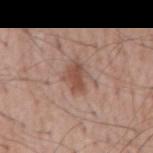Case summary:
* notes · catalogued during a skin exam; not biopsied
* body site · the back
* subject · male, approximately 60 years of age
* image-analysis metrics · an eccentricity of roughly 0.75 and a symmetry-axis asymmetry near 0.2; a lesion color around L≈50 a*≈20 b*≈27 in CIELAB, a lesion–skin lightness drop of about 10, and a lesion-to-skin contrast of about 7.5 (normalized; higher = more distinct); a border-irregularity index near 3/10 and a peripheral color-asymmetry measure near 0.5
* illumination · white-light illumination
* image · ~15 mm tile from a whole-body skin photo